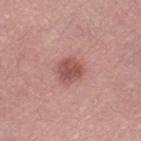Recorded during total-body skin imaging; not selected for excision or biopsy.
The total-body-photography lesion software estimated a mean CIELAB color near L≈52 a*≈25 b*≈24 and roughly 11 lightness units darker than nearby skin. And it measured an automated nevus-likeness rating near 85 out of 100 and a lesion-detection confidence of about 100/100.
The tile uses white-light illumination.
On the left thigh.
The subject is a female aged 63 to 67.
A lesion tile, about 15 mm wide, cut from a 3D total-body photograph.
About 3 mm across.